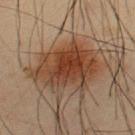Impression:
Part of a total-body skin-imaging series; this lesion was reviewed on a skin check and was not flagged for biopsy.
Clinical summary:
Measured at roughly 8 mm in maximum diameter. Located on the upper back. Cropped from a whole-body photographic skin survey; the tile spans about 15 mm. This is a cross-polarized tile. The patient is a male aged approximately 35. The lesion-visualizer software estimated an automated nevus-likeness rating near 100 out of 100.